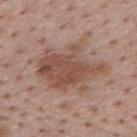| key | value |
|---|---|
| notes | total-body-photography surveillance lesion; no biopsy |
| body site | the mid back |
| image source | total-body-photography crop, ~15 mm field of view |
| illumination | white-light illumination |
| lesion diameter | ~8 mm (longest diameter) |
| patient | male, approximately 75 years of age |